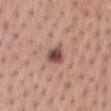A lesion tile, about 15 mm wide, cut from a 3D total-body photograph. The tile uses white-light illumination. A male subject aged 58 to 62. Located on the mid back.